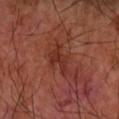This lesion was catalogued during total-body skin photography and was not selected for biopsy.
Cropped from a whole-body photographic skin survey; the tile spans about 15 mm.
The tile uses cross-polarized illumination.
A male patient aged around 70.
From the right forearm.
Approximately 4 mm at its widest.